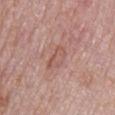The lesion was tiled from a total-body skin photograph and was not biopsied.
Cropped from a total-body skin-imaging series; the visible field is about 15 mm.
The lesion is on the mid back.
The patient is a male roughly 75 years of age.